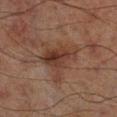workup: no biopsy performed (imaged during a skin exam); patient: male, in their 70s; location: the leg; tile lighting: cross-polarized illumination; imaging modality: ~15 mm crop, total-body skin-cancer survey; diameter: about 5.5 mm.